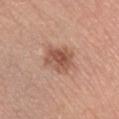The lesion was photographed on a routine skin check and not biopsied; there is no pathology result.